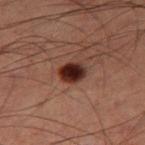The lesion was photographed on a routine skin check and not biopsied; there is no pathology result. A 15 mm close-up tile from a total-body photography series done for melanoma screening. Located on the right thigh. The subject is a male roughly 60 years of age.